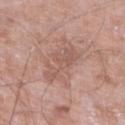Captured during whole-body skin photography for melanoma surveillance; the lesion was not biopsied. Automated image analysis of the tile measured a shape eccentricity near 0.95 and a symmetry-axis asymmetry near 0.45. The software also gave a classifier nevus-likeness of about 0/100 and a detector confidence of about 100 out of 100 that the crop contains a lesion. On the left lower leg. A close-up tile cropped from a whole-body skin photograph, about 15 mm across. About 5.5 mm across. The tile uses white-light illumination. The patient is a male approximately 75 years of age.